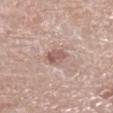No biopsy was performed on this lesion — it was imaged during a full skin examination and was not determined to be concerning. Measured at roughly 2.5 mm in maximum diameter. This is a white-light tile. The lesion-visualizer software estimated about 10 CIELAB-L* units darker than the surrounding skin and a normalized lesion–skin contrast near 6.5. A close-up tile cropped from a whole-body skin photograph, about 15 mm across. The subject is a female in their mid- to late 70s. From the left lower leg.